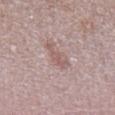workup=total-body-photography surveillance lesion; no biopsy | subject=male, about 50 years old | illumination=white-light illumination | image source=15 mm crop, total-body photography | TBP lesion metrics=an area of roughly 4 mm², a shape eccentricity near 0.95, and a symmetry-axis asymmetry near 0.5; an automated nevus-likeness rating near 5 out of 100 and lesion-presence confidence of about 90/100 | location=the left lower leg | lesion diameter=≈3.5 mm.